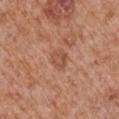Findings:
- biopsy status · imaged on a skin check; not biopsied
- diameter · ≈3 mm
- subject · male, aged approximately 65
- acquisition · total-body-photography crop, ~15 mm field of view
- anatomic site · the left upper arm
- lighting · white-light illumination